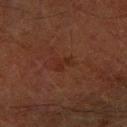workup: catalogued during a skin exam; not biopsied | imaging modality: total-body-photography crop, ~15 mm field of view | location: the right forearm | diameter: ≈2.5 mm | lighting: cross-polarized illumination | subject: male, aged 63 to 67 | automated lesion analysis: an area of roughly 3 mm², a shape eccentricity near 0.85, and a shape-asymmetry score of about 0.4 (0 = symmetric); about 4 CIELAB-L* units darker than the surrounding skin and a normalized border contrast of about 5.5; an automated nevus-likeness rating near 0 out of 100.